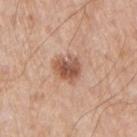Q: Is there a histopathology result?
A: catalogued during a skin exam; not biopsied
Q: How large is the lesion?
A: ≈3.5 mm
Q: Lesion location?
A: the right upper arm
Q: Who is the patient?
A: male, aged 63–67
Q: How was this image acquired?
A: ~15 mm tile from a whole-body skin photo
Q: What did automated image analysis measure?
A: an average lesion color of about L≈54 a*≈22 b*≈30 (CIELAB), about 13 CIELAB-L* units darker than the surrounding skin, and a lesion-to-skin contrast of about 9 (normalized; higher = more distinct); a border-irregularity index near 2.5/10, a color-variation rating of about 4.5/10, and radial color variation of about 1.5; an automated nevus-likeness rating near 70 out of 100 and a detector confidence of about 100 out of 100 that the crop contains a lesion
Q: How was the tile lit?
A: white-light